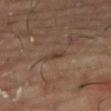  biopsy_status: not biopsied; imaged during a skin examination
  patient:
    sex: male
    age_approx: 65
  image:
    source: total-body photography crop
    field_of_view_mm: 15
  site: leg